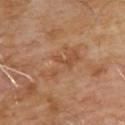* notes: no biopsy performed (imaged during a skin exam)
* subject: male, roughly 65 years of age
* location: the upper back
* tile lighting: cross-polarized
* lesion diameter: ~6.5 mm (longest diameter)
* acquisition: total-body-photography crop, ~15 mm field of view